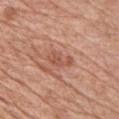Assessment: Part of a total-body skin-imaging series; this lesion was reviewed on a skin check and was not flagged for biopsy. Image and clinical context: Measured at roughly 3.5 mm in maximum diameter. Captured under white-light illumination. Located on the front of the torso. The patient is a male aged 58 to 62. A lesion tile, about 15 mm wide, cut from a 3D total-body photograph.